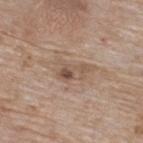Captured during whole-body skin photography for melanoma surveillance; the lesion was not biopsied.
The lesion's longest dimension is about 3.5 mm.
On the upper back.
The tile uses white-light illumination.
The patient is a female approximately 75 years of age.
A lesion tile, about 15 mm wide, cut from a 3D total-body photograph.
The total-body-photography lesion software estimated a nevus-likeness score of about 20/100 and a detector confidence of about 100 out of 100 that the crop contains a lesion.